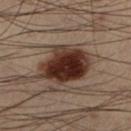Imaged during a routine full-body skin examination; the lesion was not biopsied and no histopathology is available. From the leg. Automated tile analysis of the lesion measured an average lesion color of about L≈24 a*≈15 b*≈19 (CIELAB), roughly 15 lightness units darker than nearby skin, and a normalized lesion–skin contrast near 15. And it measured lesion-presence confidence of about 100/100. A region of skin cropped from a whole-body photographic capture, roughly 15 mm wide. The patient is a male in their mid- to late 50s. Captured under cross-polarized illumination. The recorded lesion diameter is about 6 mm.